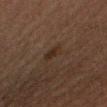* follow-up · catalogued during a skin exam; not biopsied
* tile lighting · cross-polarized illumination
* acquisition · total-body-photography crop, ~15 mm field of view
* subject · male, aged approximately 50
* site · the right upper arm